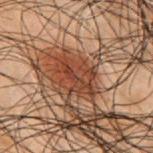Q: What did automated image analysis measure?
A: a shape eccentricity near 0.75 and a shape-asymmetry score of about 0.35 (0 = symmetric); a lesion color around L≈32 a*≈20 b*≈25 in CIELAB, a lesion–skin lightness drop of about 8, and a normalized border contrast of about 8; a classifier nevus-likeness of about 15/100 and a detector confidence of about 95 out of 100 that the crop contains a lesion
Q: How was the tile lit?
A: cross-polarized
Q: What kind of image is this?
A: total-body-photography crop, ~15 mm field of view
Q: Patient demographics?
A: male, roughly 50 years of age
Q: Where on the body is the lesion?
A: the upper back
Q: What is the lesion's diameter?
A: ≈4.5 mm